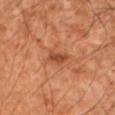The lesion was photographed on a routine skin check and not biopsied; there is no pathology result.
The lesion is on the left upper arm.
A lesion tile, about 15 mm wide, cut from a 3D total-body photograph.
Imaged with cross-polarized lighting.
Measured at roughly 3 mm in maximum diameter.
A male subject in their mid- to late 50s.